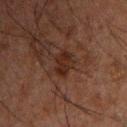Notes:
– follow-up — no biopsy performed (imaged during a skin exam)
– automated metrics — an area of roughly 4.5 mm² and two-axis asymmetry of about 0.25; roughly 6 lightness units darker than nearby skin and a normalized border contrast of about 8; a border-irregularity index near 3/10, a color-variation rating of about 2.5/10, and a peripheral color-asymmetry measure near 1; an automated nevus-likeness rating near 10 out of 100
– imaging modality — ~15 mm crop, total-body skin-cancer survey
– patient — male, in their 50s
– lesion size — about 3 mm
– lighting — cross-polarized illumination
– body site — the chest